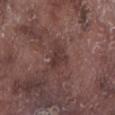<lesion>
<biopsy_status>not biopsied; imaged during a skin examination</biopsy_status>
<image>
  <source>total-body photography crop</source>
  <field_of_view_mm>15</field_of_view_mm>
</image>
<lighting>white-light</lighting>
<patient>
  <sex>male</sex>
  <age_approx>75</age_approx>
</patient>
<lesion_size>
  <long_diameter_mm_approx>3.0</long_diameter_mm_approx>
</lesion_size>
<automated_metrics>
  <border_irregularity_0_10>4.0</border_irregularity_0_10>
  <peripheral_color_asymmetry>0.5</peripheral_color_asymmetry>
  <nevus_likeness_0_100>0</nevus_likeness_0_100>
</automated_metrics>
<site>left lower leg</site>
</lesion>